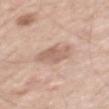The lesion was photographed on a routine skin check and not biopsied; there is no pathology result. This is a white-light tile. A 15 mm crop from a total-body photograph taken for skin-cancer surveillance. The lesion is on the arm. The subject is a male about 55 years old. The recorded lesion diameter is about 3.5 mm.